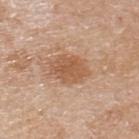follow-up: total-body-photography surveillance lesion; no biopsy | site: the upper back | subject: male, aged approximately 80 | imaging modality: ~15 mm crop, total-body skin-cancer survey | diameter: about 4.5 mm | lighting: white-light illumination.